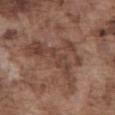The lesion was tiled from a total-body skin photograph and was not biopsied. An algorithmic analysis of the crop reported a lesion color around L≈43 a*≈19 b*≈25 in CIELAB, about 8 CIELAB-L* units darker than the surrounding skin, and a lesion-to-skin contrast of about 6.5 (normalized; higher = more distinct). It also reported a nevus-likeness score of about 0/100. The patient is a male aged approximately 75. About 8.5 mm across. From the abdomen. Cropped from a total-body skin-imaging series; the visible field is about 15 mm.